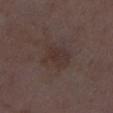Case summary:
• biopsy status — total-body-photography surveillance lesion; no biopsy
• body site — the leg
• patient — female, roughly 35 years of age
• acquisition — total-body-photography crop, ~15 mm field of view